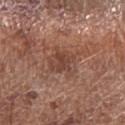Clinical impression:
This lesion was catalogued during total-body skin photography and was not selected for biopsy.
Image and clinical context:
Measured at roughly 4 mm in maximum diameter. This is a white-light tile. On the left forearm. The subject is a male roughly 70 years of age. A 15 mm close-up extracted from a 3D total-body photography capture.